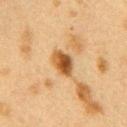The subject is a female about 40 years old.
A 15 mm crop from a total-body photograph taken for skin-cancer surveillance.
On the right upper arm.
The lesion's longest dimension is about 3.5 mm.
The tile uses cross-polarized illumination.